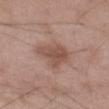Imaged during a routine full-body skin examination; the lesion was not biopsied and no histopathology is available.
Automated tile analysis of the lesion measured a border-irregularity index near 3/10, a color-variation rating of about 3/10, and a peripheral color-asymmetry measure near 1. It also reported a nevus-likeness score of about 0/100 and a detector confidence of about 100 out of 100 that the crop contains a lesion.
The lesion's longest dimension is about 4.5 mm.
Located on the left thigh.
The patient is a male aged approximately 50.
This image is a 15 mm lesion crop taken from a total-body photograph.
Imaged with white-light lighting.